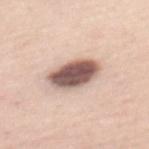No biopsy was performed on this lesion — it was imaged during a full skin examination and was not determined to be concerning.
Longest diameter approximately 5 mm.
A female subject approximately 45 years of age.
The tile uses white-light illumination.
The lesion is on the back.
Cropped from a total-body skin-imaging series; the visible field is about 15 mm.
The lesion-visualizer software estimated border irregularity of about 1.5 on a 0–10 scale, internal color variation of about 5.5 on a 0–10 scale, and a peripheral color-asymmetry measure near 1.5. It also reported an automated nevus-likeness rating near 35 out of 100 and a detector confidence of about 100 out of 100 that the crop contains a lesion.